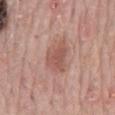workup=no biopsy performed (imaged during a skin exam) | acquisition=~15 mm crop, total-body skin-cancer survey | patient=male, roughly 70 years of age | image-analysis metrics=a lesion color around L≈54 a*≈22 b*≈25 in CIELAB, a lesion–skin lightness drop of about 9, and a normalized lesion–skin contrast near 6.5; a border-irregularity rating of about 3.5/10 and radial color variation of about 0.5 | body site=the mid back | diameter=≈4 mm.